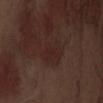Captured during whole-body skin photography for melanoma surveillance; the lesion was not biopsied. From the abdomen. This is a white-light tile. A 15 mm close-up extracted from a 3D total-body photography capture. The total-body-photography lesion software estimated an eccentricity of roughly 0.8 and a symmetry-axis asymmetry near 0.3. The software also gave a border-irregularity rating of about 3.5/10, a color-variation rating of about 1.5/10, and peripheral color asymmetry of about 0.5. The recorded lesion diameter is about 4 mm. A male subject about 70 years old.